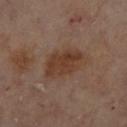biopsy status: total-body-photography surveillance lesion; no biopsy
patient: female, roughly 60 years of age
illumination: cross-polarized
site: the right lower leg
imaging modality: ~15 mm crop, total-body skin-cancer survey